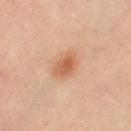Captured during whole-body skin photography for melanoma surveillance; the lesion was not biopsied.